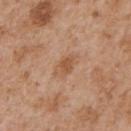The lesion was photographed on a routine skin check and not biopsied; there is no pathology result.
A lesion tile, about 15 mm wide, cut from a 3D total-body photograph.
Longest diameter approximately 3.5 mm.
The lesion is on the chest.
This is a white-light tile.
An algorithmic analysis of the crop reported an area of roughly 5.5 mm², an outline eccentricity of about 0.85 (0 = round, 1 = elongated), and a shape-asymmetry score of about 0.25 (0 = symmetric). The analysis additionally found a lesion color around L≈55 a*≈21 b*≈34 in CIELAB, a lesion–skin lightness drop of about 8, and a normalized lesion–skin contrast near 6. It also reported a border-irregularity index near 3/10, a color-variation rating of about 3/10, and a peripheral color-asymmetry measure near 1. It also reported a detector confidence of about 100 out of 100 that the crop contains a lesion.
A male patient, aged approximately 65.